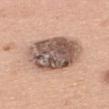– workup — no biopsy performed (imaged during a skin exam)
– image source — ~15 mm tile from a whole-body skin photo
– lesion size — ≈7.5 mm
– subject — female, roughly 60 years of age
– body site — the upper back
– tile lighting — white-light
– automated metrics — a border-irregularity index near 1/10, internal color variation of about 8 on a 0–10 scale, and radial color variation of about 2.5